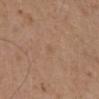The lesion was photographed on a routine skin check and not biopsied; there is no pathology result.
A 15 mm close-up extracted from a 3D total-body photography capture.
About 1.5 mm across.
The patient is a male approximately 75 years of age.
The lesion is on the chest.
Automated image analysis of the tile measured a lesion color around L≈55 a*≈17 b*≈31 in CIELAB and a normalized lesion–skin contrast near 3. The software also gave a classifier nevus-likeness of about 0/100 and a lesion-detection confidence of about 100/100.
Captured under white-light illumination.